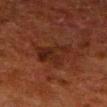Q: Is there a histopathology result?
A: no biopsy performed (imaged during a skin exam)